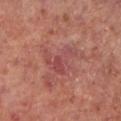Notes:
– follow-up: no biopsy performed (imaged during a skin exam)
– location: the leg
– lesion size: ~4.5 mm (longest diameter)
– patient: male, aged approximately 65
– TBP lesion metrics: an area of roughly 8.5 mm², an outline eccentricity of about 0.8 (0 = round, 1 = elongated), and two-axis asymmetry of about 0.65
– lighting: cross-polarized
– acquisition: total-body-photography crop, ~15 mm field of view